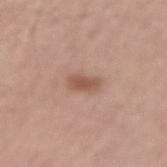workup: catalogued during a skin exam; not biopsied | subject: male, about 55 years old | imaging modality: ~15 mm crop, total-body skin-cancer survey | site: the right forearm.